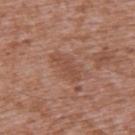| field | value |
|---|---|
| notes | no biopsy performed (imaged during a skin exam) |
| illumination | white-light |
| lesion size | ~4 mm (longest diameter) |
| anatomic site | the upper back |
| subject | male, in their mid-40s |
| imaging modality | 15 mm crop, total-body photography |